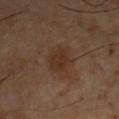Assessment: This lesion was catalogued during total-body skin photography and was not selected for biopsy. Background: Cropped from a total-body skin-imaging series; the visible field is about 15 mm. The patient is a male approximately 55 years of age. The lesion is located on the front of the torso.